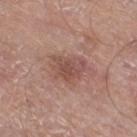Clinical impression: No biopsy was performed on this lesion — it was imaged during a full skin examination and was not determined to be concerning. Background: The patient is a male approximately 70 years of age. A region of skin cropped from a whole-body photographic capture, roughly 15 mm wide. Captured under white-light illumination. The lesion's longest dimension is about 4 mm. On the right thigh. Automated image analysis of the tile measured a lesion color around L≈50 a*≈21 b*≈24 in CIELAB, roughly 9 lightness units darker than nearby skin, and a normalized border contrast of about 6.5. And it measured a nevus-likeness score of about 0/100 and a lesion-detection confidence of about 100/100.